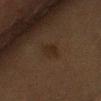Q: Was a biopsy performed?
A: imaged on a skin check; not biopsied
Q: What kind of image is this?
A: ~15 mm tile from a whole-body skin photo
Q: What did automated image analysis measure?
A: a footprint of about 3.5 mm², a shape eccentricity near 0.8, and two-axis asymmetry of about 0.3; border irregularity of about 2.5 on a 0–10 scale and a color-variation rating of about 1/10; a nevus-likeness score of about 0/100 and a detector confidence of about 100 out of 100 that the crop contains a lesion
Q: Who is the patient?
A: female, aged around 70
Q: What is the anatomic site?
A: the left upper arm